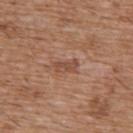Notes:
– follow-up · catalogued during a skin exam; not biopsied
– imaging modality · total-body-photography crop, ~15 mm field of view
– patient · male, approximately 60 years of age
– lighting · white-light
– body site · the chest
– image-analysis metrics · an average lesion color of about L≈48 a*≈21 b*≈30 (CIELAB) and a normalized lesion–skin contrast near 6